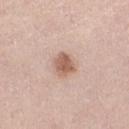notes: no biopsy performed (imaged during a skin exam); location: the left thigh; patient: female, aged approximately 65; tile lighting: white-light; acquisition: ~15 mm crop, total-body skin-cancer survey; lesion diameter: ≈3 mm.